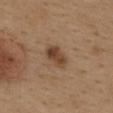No biopsy was performed on this lesion — it was imaged during a full skin examination and was not determined to be concerning.
About 3.5 mm across.
A female patient in their 40s.
Imaged with white-light lighting.
The total-body-photography lesion software estimated an area of roughly 6 mm², an eccentricity of roughly 0.8, and a shape-asymmetry score of about 0.2 (0 = symmetric). It also reported about 11 CIELAB-L* units darker than the surrounding skin and a normalized lesion–skin contrast near 8.5.
A 15 mm close-up tile from a total-body photography series done for melanoma screening.
The lesion is on the upper back.